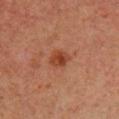Assessment:
The lesion was tiled from a total-body skin photograph and was not biopsied.
Acquisition and patient details:
A female patient, about 40 years old. This image is a 15 mm lesion crop taken from a total-body photograph. This is a cross-polarized tile. Located on the chest.